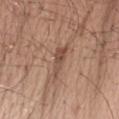| key | value |
|---|---|
| location | the left forearm |
| illumination | white-light illumination |
| image source | 15 mm crop, total-body photography |
| lesion diameter | ≈4.5 mm |
| patient | male, aged around 50 |